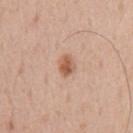• workup · imaged on a skin check; not biopsied
• lesion size · about 2.5 mm
• site · the chest
• TBP lesion metrics · an average lesion color of about L≈58 a*≈22 b*≈32 (CIELAB) and a lesion-to-skin contrast of about 8.5 (normalized; higher = more distinct); a border-irregularity index near 2.5/10, internal color variation of about 3 on a 0–10 scale, and a peripheral color-asymmetry measure near 1; a classifier nevus-likeness of about 95/100 and lesion-presence confidence of about 100/100
• illumination · white-light illumination
• image · total-body-photography crop, ~15 mm field of view
• patient · male, aged approximately 55